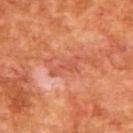notes: total-body-photography surveillance lesion; no biopsy
lighting: cross-polarized illumination
subject: male, in their mid- to late 60s
imaging modality: ~15 mm crop, total-body skin-cancer survey
diameter: about 4 mm
image-analysis metrics: a lesion area of about 4.5 mm², an outline eccentricity of about 0.95 (0 = round, 1 = elongated), and a shape-asymmetry score of about 0.45 (0 = symmetric); a nevus-likeness score of about 0/100 and a detector confidence of about 90 out of 100 that the crop contains a lesion
location: the upper back